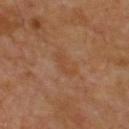notes: catalogued during a skin exam; not biopsied
patient: male, approximately 70 years of age
location: the upper back
imaging modality: total-body-photography crop, ~15 mm field of view